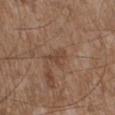No biopsy was performed on this lesion — it was imaged during a full skin examination and was not determined to be concerning.